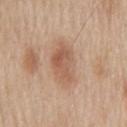{
  "patient": {
    "sex": "male",
    "age_approx": 60
  },
  "image": {
    "source": "total-body photography crop",
    "field_of_view_mm": 15
  },
  "lighting": "white-light",
  "automated_metrics": {
    "eccentricity": 0.85,
    "shape_asymmetry": 0.25,
    "vs_skin_darker_L": 10.0,
    "vs_skin_contrast_norm": 7.0,
    "border_irregularity_0_10": 3.0,
    "color_variation_0_10": 4.0,
    "peripheral_color_asymmetry": 1.5,
    "nevus_likeness_0_100": 30
  },
  "site": "mid back",
  "lesion_size": {
    "long_diameter_mm_approx": 5.5
  }
}